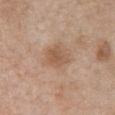Impression: No biopsy was performed on this lesion — it was imaged during a full skin examination and was not determined to be concerning. Image and clinical context: The patient is a male aged around 70. From the abdomen. The lesion's longest dimension is about 3.5 mm. Cropped from a whole-body photographic skin survey; the tile spans about 15 mm. This is a white-light tile.